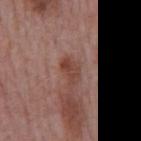follow-up = total-body-photography surveillance lesion; no biopsy.